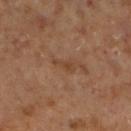This lesion was catalogued during total-body skin photography and was not selected for biopsy. A 15 mm close-up tile from a total-body photography series done for melanoma screening. The lesion is located on the leg. The tile uses cross-polarized illumination. A male patient, approximately 60 years of age. About 3 mm across.